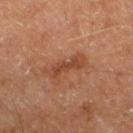Clinical impression: The lesion was photographed on a routine skin check and not biopsied; there is no pathology result. Background: The subject is a male in their 60s. Located on the right leg. An algorithmic analysis of the crop reported an eccentricity of roughly 0.95 and two-axis asymmetry of about 0.25. It also reported an average lesion color of about L≈43 a*≈24 b*≈32 (CIELAB), a lesion–skin lightness drop of about 8, and a lesion-to-skin contrast of about 7 (normalized; higher = more distinct). Cropped from a whole-body photographic skin survey; the tile spans about 15 mm. The recorded lesion diameter is about 5 mm. This is a cross-polarized tile.